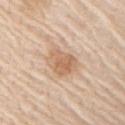notes = total-body-photography surveillance lesion; no biopsy | patient = male, aged 78 to 82 | image = 15 mm crop, total-body photography | anatomic site = the right upper arm | lesion diameter = ≈4 mm | automated lesion analysis = a footprint of about 9 mm² and a symmetry-axis asymmetry near 0.2; a normalized border contrast of about 7; a classifier nevus-likeness of about 75/100.